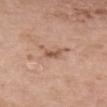Clinical summary: The patient is a female about 40 years old. The lesion's longest dimension is about 2 mm. Automated image analysis of the tile measured an area of roughly 2.5 mm², an outline eccentricity of about 0.8 (0 = round, 1 = elongated), and a symmetry-axis asymmetry near 0.35. And it measured an automated nevus-likeness rating near 0 out of 100 and a lesion-detection confidence of about 100/100. From the chest. A region of skin cropped from a whole-body photographic capture, roughly 15 mm wide. The tile uses white-light illumination.